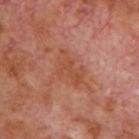This lesion was catalogued during total-body skin photography and was not selected for biopsy.
The tile uses cross-polarized illumination.
A 15 mm close-up tile from a total-body photography series done for melanoma screening.
The lesion is located on the back.
Measured at roughly 4.5 mm in maximum diameter.
A male patient in their 70s.
The lesion-visualizer software estimated an area of roughly 8.5 mm² and two-axis asymmetry of about 0.45. And it measured a lesion color around L≈48 a*≈26 b*≈32 in CIELAB, a lesion–skin lightness drop of about 6, and a lesion-to-skin contrast of about 6 (normalized; higher = more distinct). The software also gave a border-irregularity rating of about 5.5/10 and peripheral color asymmetry of about 1.